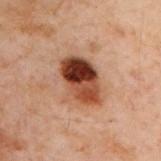notes = total-body-photography surveillance lesion; no biopsy
subject = male, about 50 years old
site = the upper back
image source = total-body-photography crop, ~15 mm field of view